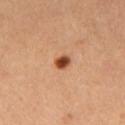| feature | finding |
|---|---|
| workup | imaged on a skin check; not biopsied |
| image source | total-body-photography crop, ~15 mm field of view |
| automated lesion analysis | an automated nevus-likeness rating near 100 out of 100 and a lesion-detection confidence of about 100/100 |
| tile lighting | cross-polarized |
| lesion diameter | ~2 mm (longest diameter) |
| subject | female, aged 28–32 |
| site | the leg |